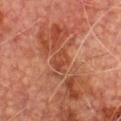notes = no biopsy performed (imaged during a skin exam) | patient = male, in their mid-70s | image = ~15 mm tile from a whole-body skin photo | illumination = cross-polarized | anatomic site = the chest.